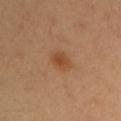The lesion was tiled from a total-body skin photograph and was not biopsied.
A region of skin cropped from a whole-body photographic capture, roughly 15 mm wide.
The lesion is located on the left upper arm.
A female subject, about 35 years old.